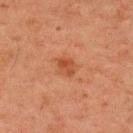Image and clinical context:
Captured under cross-polarized illumination. This image is a 15 mm lesion crop taken from a total-body photograph. A male subject, in their 60s. From the upper back.